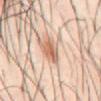Impression: Captured during whole-body skin photography for melanoma surveillance; the lesion was not biopsied. Image and clinical context: A 15 mm crop from a total-body photograph taken for skin-cancer surveillance. Approximately 3.5 mm at its widest. A male patient about 40 years old. The lesion is located on the abdomen.